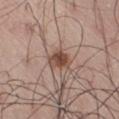Impression: Part of a total-body skin-imaging series; this lesion was reviewed on a skin check and was not flagged for biopsy. Context: Approximately 3 mm at its widest. Captured under white-light illumination. Located on the lower back. A male patient, about 70 years old. A region of skin cropped from a whole-body photographic capture, roughly 15 mm wide.